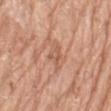Assessment:
No biopsy was performed on this lesion — it was imaged during a full skin examination and was not determined to be concerning.
Background:
Approximately 3 mm at its widest. A female patient aged around 75. Captured under white-light illumination. A lesion tile, about 15 mm wide, cut from a 3D total-body photograph. The total-body-photography lesion software estimated an outline eccentricity of about 0.85 (0 = round, 1 = elongated) and a shape-asymmetry score of about 0.45 (0 = symmetric). The lesion is on the left thigh.